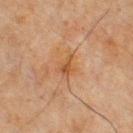No biopsy was performed on this lesion — it was imaged during a full skin examination and was not determined to be concerning.
The lesion is located on the chest.
Captured under cross-polarized illumination.
A male patient, approximately 65 years of age.
Approximately 2.5 mm at its widest.
This image is a 15 mm lesion crop taken from a total-body photograph.
The lesion-visualizer software estimated a border-irregularity rating of about 4.5/10 and internal color variation of about 2.5 on a 0–10 scale.